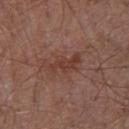Q: Was this lesion biopsied?
A: catalogued during a skin exam; not biopsied
Q: What kind of image is this?
A: ~15 mm crop, total-body skin-cancer survey
Q: Where on the body is the lesion?
A: the chest
Q: Lesion size?
A: ≈5 mm
Q: Who is the patient?
A: male, about 55 years old